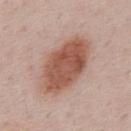workup: catalogued during a skin exam; not biopsied | image: 15 mm crop, total-body photography | body site: the mid back | subject: male, roughly 50 years of age.